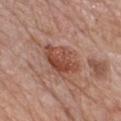This lesion was catalogued during total-body skin photography and was not selected for biopsy. Cropped from a total-body skin-imaging series; the visible field is about 15 mm. Approximately 5 mm at its widest. A female patient aged approximately 70. Automated image analysis of the tile measured a footprint of about 11 mm², an outline eccentricity of about 0.85 (0 = round, 1 = elongated), and two-axis asymmetry of about 0.3. From the chest. The tile uses white-light illumination.